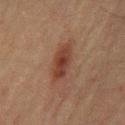Impression:
Part of a total-body skin-imaging series; this lesion was reviewed on a skin check and was not flagged for biopsy.
Context:
This is a cross-polarized tile. The subject is a male aged approximately 60. About 6 mm across. Cropped from a whole-body photographic skin survey; the tile spans about 15 mm. The lesion is located on the front of the torso.